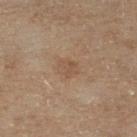{"biopsy_status": "not biopsied; imaged during a skin examination", "automated_metrics": {"cielab_L": 37, "cielab_a": 12, "cielab_b": 23, "vs_skin_darker_L": 5.0, "vs_skin_contrast_norm": 4.5, "border_irregularity_0_10": 1.5, "color_variation_0_10": 2.0, "peripheral_color_asymmetry": 0.5}, "lighting": "cross-polarized", "patient": {"sex": "male", "age_approx": 70}, "image": {"source": "total-body photography crop", "field_of_view_mm": 15}, "site": "right lower leg"}